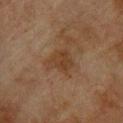  biopsy_status: not biopsied; imaged during a skin examination
  image:
    source: total-body photography crop
    field_of_view_mm: 15
  lesion_size:
    long_diameter_mm_approx: 3.5
  patient:
    sex: male
    age_approx: 75
  site: upper back
  lighting: cross-polarized
  automated_metrics:
    eccentricity: 0.65
    shape_asymmetry: 0.35
    border_irregularity_0_10: 3.5
    nevus_likeness_0_100: 0
    lesion_detection_confidence_0_100: 100